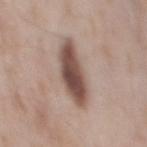Findings:
– workup · total-body-photography surveillance lesion; no biopsy
– diameter · about 6.5 mm
– image source · 15 mm crop, total-body photography
– automated lesion analysis · an area of roughly 15 mm²; border irregularity of about 2.5 on a 0–10 scale, internal color variation of about 5 on a 0–10 scale, and a peripheral color-asymmetry measure near 1.5; a nevus-likeness score of about 100/100 and a lesion-detection confidence of about 100/100
– body site · the mid back
– patient · male, aged 53–57
– lighting · white-light